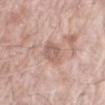Notes:
* workup · total-body-photography surveillance lesion; no biopsy
* subject · male, aged around 75
* tile lighting · white-light illumination
* acquisition · ~15 mm tile from a whole-body skin photo
* site · the left forearm
* diameter · about 3.5 mm